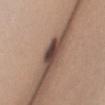follow-up: imaged on a skin check; not biopsied
image source: ~15 mm tile from a whole-body skin photo
image-analysis metrics: an outline eccentricity of about 0.9 (0 = round, 1 = elongated) and a shape-asymmetry score of about 0.25 (0 = symmetric); an average lesion color of about L≈40 a*≈17 b*≈21 (CIELAB), a lesion–skin lightness drop of about 18, and a lesion-to-skin contrast of about 14 (normalized; higher = more distinct); border irregularity of about 3 on a 0–10 scale and a within-lesion color-variation index near 3/10; a nevus-likeness score of about 20/100 and lesion-presence confidence of about 0/100
diameter: ≈3.5 mm
patient: female, roughly 25 years of age
lighting: white-light
body site: the chest